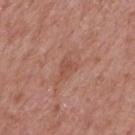– notes — catalogued during a skin exam; not biopsied
– patient — male, aged approximately 65
– imaging modality — ~15 mm tile from a whole-body skin photo
– body site — the mid back
– automated metrics — a classifier nevus-likeness of about 0/100 and a detector confidence of about 100 out of 100 that the crop contains a lesion
– illumination — white-light